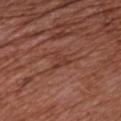workup: no biopsy performed (imaged during a skin exam) | imaging modality: 15 mm crop, total-body photography | TBP lesion metrics: an area of roughly 3.5 mm² and a symmetry-axis asymmetry near 0.6; a normalized border contrast of about 5.5 | size: about 2.5 mm | illumination: white-light | subject: male, aged 73–77 | site: the chest.